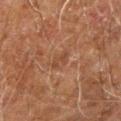Recorded during total-body skin imaging; not selected for excision or biopsy. Automated image analysis of the tile measured border irregularity of about 5 on a 0–10 scale, a color-variation rating of about 0/10, and peripheral color asymmetry of about 0. The analysis additionally found a nevus-likeness score of about 0/100 and lesion-presence confidence of about 95/100. The tile uses cross-polarized illumination. The patient is a male aged approximately 60. The lesion is on the left arm. A roughly 15 mm field-of-view crop from a total-body skin photograph. Measured at roughly 2.5 mm in maximum diameter.